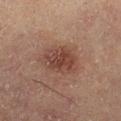Clinical impression:
The lesion was tiled from a total-body skin photograph and was not biopsied.
Acquisition and patient details:
This is a cross-polarized tile. This image is a 15 mm lesion crop taken from a total-body photograph. On the right lower leg. The lesion's longest dimension is about 5 mm. A male patient about 60 years old.